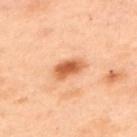Clinical impression:
No biopsy was performed on this lesion — it was imaged during a full skin examination and was not determined to be concerning.
Acquisition and patient details:
A 15 mm crop from a total-body photograph taken for skin-cancer surveillance. The recorded lesion diameter is about 3.5 mm. The tile uses cross-polarized illumination. Located on the upper back. A female subject aged around 55.